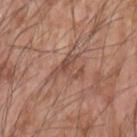Clinical impression:
The lesion was tiled from a total-body skin photograph and was not biopsied.
Context:
The lesion-visualizer software estimated an average lesion color of about L≈49 a*≈21 b*≈27 (CIELAB) and about 9 CIELAB-L* units darker than the surrounding skin. It also reported a border-irregularity rating of about 9/10 and radial color variation of about 1. And it measured a nevus-likeness score of about 0/100 and lesion-presence confidence of about 80/100. The lesion's longest dimension is about 4 mm. A male patient aged 58 to 62. Captured under white-light illumination. Cropped from a whole-body photographic skin survey; the tile spans about 15 mm. Located on the left forearm.